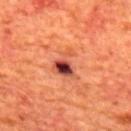Clinical impression:
Imaged during a routine full-body skin examination; the lesion was not biopsied and no histopathology is available.
Clinical summary:
This is a cross-polarized tile. The lesion is on the upper back. The subject is a male about 65 years old. A close-up tile cropped from a whole-body skin photograph, about 15 mm across. Longest diameter approximately 3.5 mm. Automated image analysis of the tile measured an area of roughly 7.5 mm² and an eccentricity of roughly 0.6. The analysis additionally found a classifier nevus-likeness of about 45/100.